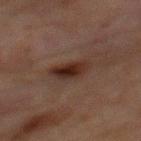Imaged during a routine full-body skin examination; the lesion was not biopsied and no histopathology is available.
A male subject about 65 years old.
Imaged with cross-polarized lighting.
A close-up tile cropped from a whole-body skin photograph, about 15 mm across.
About 4 mm across.
On the chest.